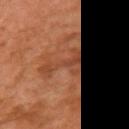{"biopsy_status": "not biopsied; imaged during a skin examination", "site": "right upper arm", "lighting": "cross-polarized", "patient": {"sex": "female", "age_approx": 60}, "lesion_size": {"long_diameter_mm_approx": 4.5}, "image": {"source": "total-body photography crop", "field_of_view_mm": 15}}